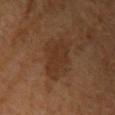workup=imaged on a skin check; not biopsied
acquisition=15 mm crop, total-body photography
patient=female, aged 58–62
illumination=cross-polarized
size=about 5.5 mm
TBP lesion metrics=a lesion area of about 14 mm²; a border-irregularity index near 3.5/10, internal color variation of about 2.5 on a 0–10 scale, and peripheral color asymmetry of about 1
location=the upper back